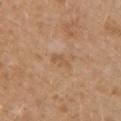Imaged during a routine full-body skin examination; the lesion was not biopsied and no histopathology is available. On the left upper arm. A region of skin cropped from a whole-body photographic capture, roughly 15 mm wide. A female subject approximately 30 years of age.